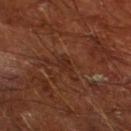Assessment: Imaged during a routine full-body skin examination; the lesion was not biopsied and no histopathology is available. Clinical summary: A male subject, about 65 years old. Approximately 2.5 mm at its widest. A roughly 15 mm field-of-view crop from a total-body skin photograph. Located on the left lower leg.